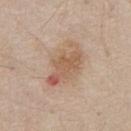No biopsy was performed on this lesion — it was imaged during a full skin examination and was not determined to be concerning. A roughly 15 mm field-of-view crop from a total-body skin photograph. Automated tile analysis of the lesion measured a symmetry-axis asymmetry near 0.3. And it measured border irregularity of about 5 on a 0–10 scale, a color-variation rating of about 5/10, and a peripheral color-asymmetry measure near 1. The software also gave an automated nevus-likeness rating near 55 out of 100. The tile uses white-light illumination. A male patient approximately 80 years of age. Located on the front of the torso. The lesion's longest dimension is about 5.5 mm.